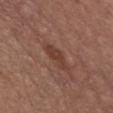* notes — total-body-photography surveillance lesion; no biopsy